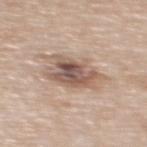Clinical impression:
No biopsy was performed on this lesion — it was imaged during a full skin examination and was not determined to be concerning.
Image and clinical context:
The lesion-visualizer software estimated a footprint of about 14 mm², an eccentricity of roughly 0.75, and two-axis asymmetry of about 0.25. It also reported a lesion-detection confidence of about 100/100. Imaged with white-light lighting. Longest diameter approximately 5.5 mm. A lesion tile, about 15 mm wide, cut from a 3D total-body photograph. The subject is a male aged approximately 70. The lesion is located on the upper back.